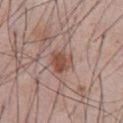Assessment: Captured during whole-body skin photography for melanoma surveillance; the lesion was not biopsied. Clinical summary: A 15 mm crop from a total-body photograph taken for skin-cancer surveillance. The lesion is on the front of the torso. This is a white-light tile. Measured at roughly 3 mm in maximum diameter. A male subject aged around 55.